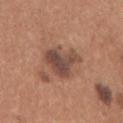| key | value |
|---|---|
| follow-up | total-body-photography surveillance lesion; no biopsy |
| subject | female, aged 18–22 |
| acquisition | ~15 mm tile from a whole-body skin photo |
| location | the right upper arm |
| lesion diameter | ≈5 mm |
| TBP lesion metrics | an eccentricity of roughly 0.65 and two-axis asymmetry of about 0.35; a classifier nevus-likeness of about 10/100 and a lesion-detection confidence of about 100/100 |